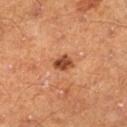notes = no biopsy performed (imaged during a skin exam)
tile lighting = cross-polarized
patient = male, in their 60s
lesion diameter = ~2.5 mm (longest diameter)
image source = total-body-photography crop, ~15 mm field of view
automated metrics = border irregularity of about 2.5 on a 0–10 scale and internal color variation of about 4 on a 0–10 scale; an automated nevus-likeness rating near 80 out of 100 and lesion-presence confidence of about 100/100
body site = the left lower leg